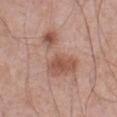<case>
  <biopsy_status>not biopsied; imaged during a skin examination</biopsy_status>
  <site>abdomen</site>
  <lighting>white-light</lighting>
  <automated_metrics>
    <area_mm2_approx>22.0</area_mm2_approx>
    <eccentricity>0.85</eccentricity>
    <shape_asymmetry>0.5</shape_asymmetry>
    <color_variation_0_10>7.5</color_variation_0_10>
    <peripheral_color_asymmetry>2.5</peripheral_color_asymmetry>
  </automated_metrics>
  <patient>
    <sex>male</sex>
    <age_approx>70</age_approx>
  </patient>
  <lesion_size>
    <long_diameter_mm_approx>8.0</long_diameter_mm_approx>
  </lesion_size>
  <image>
    <source>total-body photography crop</source>
    <field_of_view_mm>15</field_of_view_mm>
  </image>
</case>